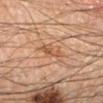This lesion was catalogued during total-body skin photography and was not selected for biopsy.
The patient is a male approximately 45 years of age.
The lesion is on the right forearm.
A 15 mm close-up tile from a total-body photography series done for melanoma screening.